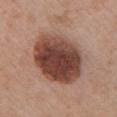Part of a total-body skin-imaging series; this lesion was reviewed on a skin check and was not flagged for biopsy. A female patient roughly 40 years of age. This is a white-light tile. On the chest. The recorded lesion diameter is about 7.5 mm. A 15 mm close-up tile from a total-body photography series done for melanoma screening.